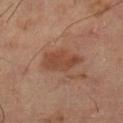biopsy status — no biopsy performed (imaged during a skin exam) | patient — female, about 60 years old | automated metrics — an area of roughly 10 mm², an eccentricity of roughly 0.75, and a shape-asymmetry score of about 0.2 (0 = symmetric); a nevus-likeness score of about 65/100 | image — ~15 mm tile from a whole-body skin photo | anatomic site — the right leg | illumination — cross-polarized illumination.